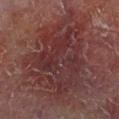| key | value |
|---|---|
| workup | catalogued during a skin exam; not biopsied |
| image | total-body-photography crop, ~15 mm field of view |
| body site | the right lower leg |
| patient | male, approximately 60 years of age |